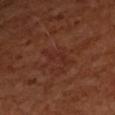| field | value |
|---|---|
| biopsy status | imaged on a skin check; not biopsied |
| body site | the left forearm |
| patient | female, in their 50s |
| imaging modality | total-body-photography crop, ~15 mm field of view |
| lighting | cross-polarized |
| TBP lesion metrics | an area of roughly 5 mm², an outline eccentricity of about 0.9 (0 = round, 1 = elongated), and a shape-asymmetry score of about 0.3 (0 = symmetric); an automated nevus-likeness rating near 0 out of 100 and a lesion-detection confidence of about 100/100 |